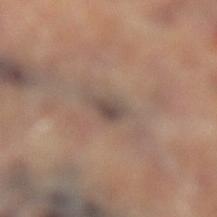This lesion was catalogued during total-body skin photography and was not selected for biopsy. Automated tile analysis of the lesion measured an automated nevus-likeness rating near 0 out of 100 and a lesion-detection confidence of about 60/100. The lesion is located on the leg. A 15 mm close-up extracted from a 3D total-body photography capture. This is a cross-polarized tile. Approximately 3 mm at its widest.